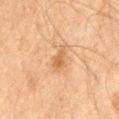The lesion was tiled from a total-body skin photograph and was not biopsied.
Cropped from a total-body skin-imaging series; the visible field is about 15 mm.
On the chest.
A male subject, in their 60s.